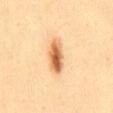  biopsy_status: not biopsied; imaged during a skin examination
  patient:
    sex: male
    age_approx: 35
  image:
    source: total-body photography crop
    field_of_view_mm: 15
  site: mid back
  automated_metrics:
    eccentricity: 0.9
    shape_asymmetry: 0.2
    cielab_L: 59
    cielab_a: 22
    cielab_b: 39
    vs_skin_darker_L: 15.0
    vs_skin_contrast_norm: 10.0
    border_irregularity_0_10: 2.5
    color_variation_0_10: 7.5
    peripheral_color_asymmetry: 2.5
    nevus_likeness_0_100: 100
  lighting: cross-polarized